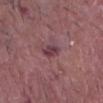Assessment: Part of a total-body skin-imaging series; this lesion was reviewed on a skin check and was not flagged for biopsy. Image and clinical context: Imaged with white-light lighting. On the head or neck. The subject is a male aged around 85 or older. Automated tile analysis of the lesion measured an area of roughly 4 mm², an outline eccentricity of about 0.75 (0 = round, 1 = elongated), and a symmetry-axis asymmetry near 0.2. The analysis additionally found an average lesion color of about L≈39 a*≈24 b*≈13 (CIELAB), a lesion–skin lightness drop of about 10, and a normalized border contrast of about 8.5. The recorded lesion diameter is about 2.5 mm. Cropped from a whole-body photographic skin survey; the tile spans about 15 mm.